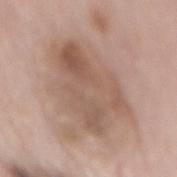Clinical impression:
No biopsy was performed on this lesion — it was imaged during a full skin examination and was not determined to be concerning.
Clinical summary:
Cropped from a total-body skin-imaging series; the visible field is about 15 mm. The patient is a female in their mid-60s. On the mid back. Approximately 9 mm at its widest. Automated image analysis of the tile measured an area of roughly 33 mm², a shape eccentricity near 0.8, and a shape-asymmetry score of about 0.3 (0 = symmetric). The software also gave a lesion color around L≈56 a*≈17 b*≈26 in CIELAB, a lesion–skin lightness drop of about 10, and a lesion-to-skin contrast of about 6.5 (normalized; higher = more distinct). The analysis additionally found internal color variation of about 5.5 on a 0–10 scale and radial color variation of about 2. The analysis additionally found a nevus-likeness score of about 0/100. Imaged with white-light lighting.